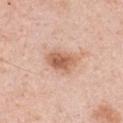Findings:
• follow-up · catalogued during a skin exam; not biopsied
• tile lighting · white-light
• imaging modality · ~15 mm crop, total-body skin-cancer survey
• subject · male, approximately 50 years of age
• size · ≈4 mm
• image-analysis metrics · a footprint of about 8.5 mm², an eccentricity of roughly 0.75, and two-axis asymmetry of about 0.25; an average lesion color of about L≈62 a*≈22 b*≈32 (CIELAB), roughly 12 lightness units darker than nearby skin, and a normalized border contrast of about 8; a nevus-likeness score of about 85/100
• body site · the front of the torso